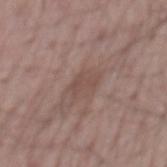| key | value |
|---|---|
| biopsy status | catalogued during a skin exam; not biopsied |
| lighting | white-light |
| size | ~3 mm (longest diameter) |
| site | the mid back |
| subject | male, roughly 65 years of age |
| image source | ~15 mm crop, total-body skin-cancer survey |